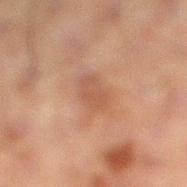Assessment:
No biopsy was performed on this lesion — it was imaged during a full skin examination and was not determined to be concerning.
Acquisition and patient details:
Automated tile analysis of the lesion measured border irregularity of about 2 on a 0–10 scale, internal color variation of about 2.5 on a 0–10 scale, and a peripheral color-asymmetry measure near 1. And it measured an automated nevus-likeness rating near 0 out of 100 and lesion-presence confidence of about 100/100. Cropped from a total-body skin-imaging series; the visible field is about 15 mm. The patient is a male aged approximately 60. The recorded lesion diameter is about 3.5 mm. Located on the left leg.